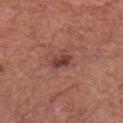No biopsy was performed on this lesion — it was imaged during a full skin examination and was not determined to be concerning.
A male subject, aged 73 to 77.
An algorithmic analysis of the crop reported an average lesion color of about L≈40 a*≈23 b*≈23 (CIELAB) and a lesion-to-skin contrast of about 9 (normalized; higher = more distinct). The analysis additionally found an automated nevus-likeness rating near 45 out of 100 and a lesion-detection confidence of about 100/100.
The tile uses white-light illumination.
Cropped from a whole-body photographic skin survey; the tile spans about 15 mm.
The lesion is located on the chest.